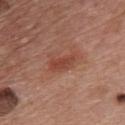  image:
    source: total-body photography crop
    field_of_view_mm: 15
  patient:
    sex: male
    age_approx: 65
  lesion_size:
    long_diameter_mm_approx: 4.0
  site: front of the torso